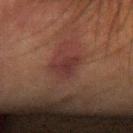Q: Is there a histopathology result?
A: imaged on a skin check; not biopsied
Q: What are the patient's age and sex?
A: male, approximately 80 years of age
Q: What is the imaging modality?
A: 15 mm crop, total-body photography
Q: Lesion size?
A: ~3.5 mm (longest diameter)
Q: What is the anatomic site?
A: the left forearm